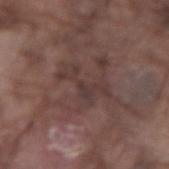Captured during whole-body skin photography for melanoma surveillance; the lesion was not biopsied. On the right forearm. Cropped from a whole-body photographic skin survey; the tile spans about 15 mm. Measured at roughly 5.5 mm in maximum diameter. The subject is a male approximately 75 years of age. The lesion-visualizer software estimated border irregularity of about 9.5 on a 0–10 scale and a color-variation rating of about 2.5/10. And it measured a nevus-likeness score of about 0/100 and a detector confidence of about 55 out of 100 that the crop contains a lesion.